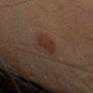Impression: This lesion was catalogued during total-body skin photography and was not selected for biopsy. Context: The recorded lesion diameter is about 3.5 mm. On the left arm. A male subject, roughly 50 years of age. A region of skin cropped from a whole-body photographic capture, roughly 15 mm wide. Captured under cross-polarized illumination. An algorithmic analysis of the crop reported a mean CIELAB color near L≈23 a*≈14 b*≈20, a lesion–skin lightness drop of about 5, and a lesion-to-skin contrast of about 6.5 (normalized; higher = more distinct). The software also gave a nevus-likeness score of about 90/100.